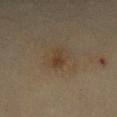Imaged during a routine full-body skin examination; the lesion was not biopsied and no histopathology is available. Located on the abdomen. A 15 mm close-up tile from a total-body photography series done for melanoma screening. A female patient, approximately 60 years of age.